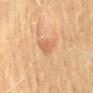notes: imaged on a skin check; not biopsied | diameter: about 3.5 mm | image-analysis metrics: an area of roughly 5 mm², a shape eccentricity near 0.85, and two-axis asymmetry of about 0.35; a nevus-likeness score of about 95/100 | patient: female, approximately 55 years of age | body site: the mid back | illumination: cross-polarized illumination | imaging modality: ~15 mm crop, total-body skin-cancer survey.